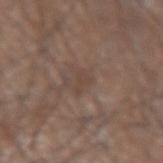* workup · total-body-photography surveillance lesion; no biopsy
* patient · male, in their 60s
* site · the left upper arm
* acquisition · ~15 mm crop, total-body skin-cancer survey